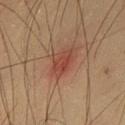Assessment:
The lesion was photographed on a routine skin check and not biopsied; there is no pathology result.
Clinical summary:
A roughly 15 mm field-of-view crop from a total-body skin photograph. Approximately 4 mm at its widest. The patient is a male aged around 35. On the leg. Captured under cross-polarized illumination.